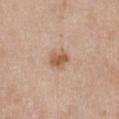{"patient": {"sex": "female", "age_approx": 40}, "lighting": "white-light", "lesion_size": {"long_diameter_mm_approx": 2.5}, "automated_metrics": {"area_mm2_approx": 4.5, "eccentricity": 0.65, "shape_asymmetry": 0.25, "cielab_L": 57, "cielab_a": 20, "cielab_b": 33, "vs_skin_darker_L": 11.0, "vs_skin_contrast_norm": 8.5, "nevus_likeness_0_100": 75}, "image": {"source": "total-body photography crop", "field_of_view_mm": 15}, "site": "chest"}